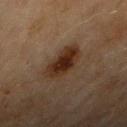follow-up: no biopsy performed (imaged during a skin exam); lesion size: ~5 mm (longest diameter); image source: ~15 mm tile from a whole-body skin photo; patient: female, approximately 70 years of age; anatomic site: the arm; tile lighting: cross-polarized illumination.